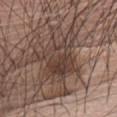{"biopsy_status": "not biopsied; imaged during a skin examination", "patient": {"sex": "male", "age_approx": 75}, "image": {"source": "total-body photography crop", "field_of_view_mm": 15}, "site": "chest", "lighting": "white-light"}